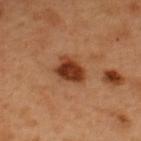The lesion was photographed on a routine skin check and not biopsied; there is no pathology result.
A male subject approximately 50 years of age.
A close-up tile cropped from a whole-body skin photograph, about 15 mm across.
On the upper back.